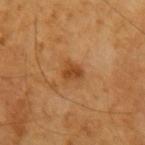No biopsy was performed on this lesion — it was imaged during a full skin examination and was not determined to be concerning.
On the right upper arm.
The tile uses cross-polarized illumination.
Cropped from a whole-body photographic skin survey; the tile spans about 15 mm.
The patient is a male roughly 60 years of age.